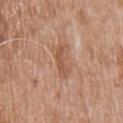Recorded during total-body skin imaging; not selected for excision or biopsy. Cropped from a whole-body photographic skin survey; the tile spans about 15 mm. The subject is a male approximately 80 years of age. The lesion is located on the upper back. Captured under white-light illumination. The recorded lesion diameter is about 3.5 mm.